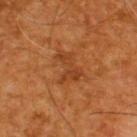Recorded during total-body skin imaging; not selected for excision or biopsy. About 3.5 mm across. The total-body-photography lesion software estimated a lesion area of about 6 mm² and an outline eccentricity of about 0.8 (0 = round, 1 = elongated). The analysis additionally found a border-irregularity index near 6.5/10 and internal color variation of about 1.5 on a 0–10 scale. The analysis additionally found a nevus-likeness score of about 0/100 and a detector confidence of about 100 out of 100 that the crop contains a lesion. A male subject roughly 60 years of age. From the back. A lesion tile, about 15 mm wide, cut from a 3D total-body photograph.